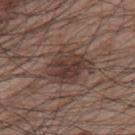Clinical impression:
Recorded during total-body skin imaging; not selected for excision or biopsy.
Acquisition and patient details:
Cropped from a total-body skin-imaging series; the visible field is about 15 mm. A male subject aged 63–67. The lesion is located on the back.